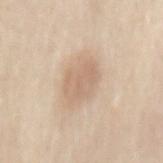Impression:
This lesion was catalogued during total-body skin photography and was not selected for biopsy.
Background:
Located on the mid back. Imaged with white-light lighting. The recorded lesion diameter is about 4 mm. A region of skin cropped from a whole-body photographic capture, roughly 15 mm wide. A female subject aged around 70.